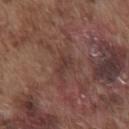  patient:
    sex: male
    age_approx: 75
  image:
    source: total-body photography crop
    field_of_view_mm: 15
  automated_metrics:
    area_mm2_approx: 2.5
    eccentricity: 0.85
    cielab_L: 36
    cielab_a: 19
    cielab_b: 21
    vs_skin_darker_L: 6.0
    vs_skin_contrast_norm: 6.0
    nevus_likeness_0_100: 0
    lesion_detection_confidence_0_100: 90
  lighting: white-light
  site: chest